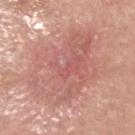<tbp_lesion>
<biopsy_status>not biopsied; imaged during a skin examination</biopsy_status>
<site>head or neck</site>
<image>
  <source>total-body photography crop</source>
  <field_of_view_mm>15</field_of_view_mm>
</image>
<patient>
  <sex>male</sex>
  <age_approx>70</age_approx>
</patient>
<lesion_size>
  <long_diameter_mm_approx>10.5</long_diameter_mm_approx>
</lesion_size>
</tbp_lesion>